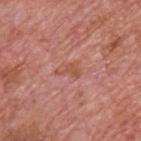The lesion was photographed on a routine skin check and not biopsied; there is no pathology result.
The lesion is located on the back.
A 15 mm close-up extracted from a 3D total-body photography capture.
A male subject in their mid-70s.